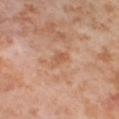<tbp_lesion>
<biopsy_status>not biopsied; imaged during a skin examination</biopsy_status>
<lighting>cross-polarized</lighting>
<automated_metrics>
  <area_mm2_approx>4.0</area_mm2_approx>
  <eccentricity>0.9</eccentricity>
  <cielab_L>58</cielab_L>
  <cielab_a>23</cielab_a>
  <cielab_b>34</cielab_b>
  <vs_skin_darker_L>6.0</vs_skin_darker_L>
  <vs_skin_contrast_norm>4.5</vs_skin_contrast_norm>
  <border_irregularity_0_10>3.0</border_irregularity_0_10>
  <color_variation_0_10>2.5</color_variation_0_10>
  <peripheral_color_asymmetry>1.0</peripheral_color_asymmetry>
</automated_metrics>
<image>
  <source>total-body photography crop</source>
  <field_of_view_mm>15</field_of_view_mm>
</image>
<site>right thigh</site>
<lesion_size>
  <long_diameter_mm_approx>3.5</long_diameter_mm_approx>
</lesion_size>
<patient>
  <sex>female</sex>
  <age_approx>55</age_approx>
</patient>
</tbp_lesion>